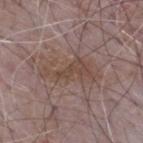This lesion was catalogued during total-body skin photography and was not selected for biopsy. The lesion's longest dimension is about 6.5 mm. From the mid back. A male subject, roughly 65 years of age. A 15 mm crop from a total-body photograph taken for skin-cancer surveillance. The tile uses white-light illumination. Automated image analysis of the tile measured a border-irregularity index near 8/10, a within-lesion color-variation index near 2.5/10, and peripheral color asymmetry of about 0.5.